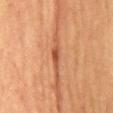Imaged during a routine full-body skin examination; the lesion was not biopsied and no histopathology is available. Cropped from a total-body skin-imaging series; the visible field is about 15 mm. An algorithmic analysis of the crop reported an average lesion color of about L≈42 a*≈23 b*≈31 (CIELAB), roughly 10 lightness units darker than nearby skin, and a lesion-to-skin contrast of about 7.5 (normalized; higher = more distinct). The analysis additionally found an automated nevus-likeness rating near 20 out of 100 and a detector confidence of about 65 out of 100 that the crop contains a lesion. From the back. About 3 mm across. A female patient, aged around 60. Captured under cross-polarized illumination.